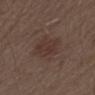Case summary:
- notes — imaged on a skin check; not biopsied
- subject — male, aged approximately 70
- image — ~15 mm crop, total-body skin-cancer survey
- location — the left thigh